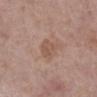Imaged during a routine full-body skin examination; the lesion was not biopsied and no histopathology is available.
A region of skin cropped from a whole-body photographic capture, roughly 15 mm wide.
The recorded lesion diameter is about 2.5 mm.
From the right lower leg.
The lesion-visualizer software estimated a mean CIELAB color near L≈53 a*≈19 b*≈27 and a lesion-to-skin contrast of about 5.5 (normalized; higher = more distinct). And it measured border irregularity of about 1.5 on a 0–10 scale, internal color variation of about 2 on a 0–10 scale, and peripheral color asymmetry of about 1. It also reported an automated nevus-likeness rating near 0 out of 100 and a lesion-detection confidence of about 100/100.
The patient is a female about 65 years old.